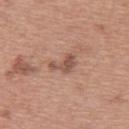Assessment:
Imaged during a routine full-body skin examination; the lesion was not biopsied and no histopathology is available.
Acquisition and patient details:
On the upper back. The subject is a female aged 58–62. This image is a 15 mm lesion crop taken from a total-body photograph.